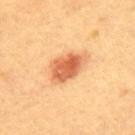The lesion was photographed on a routine skin check and not biopsied; there is no pathology result.
The recorded lesion diameter is about 5 mm.
Imaged with cross-polarized lighting.
A female subject, roughly 60 years of age.
The lesion is on the mid back.
A lesion tile, about 15 mm wide, cut from a 3D total-body photograph.